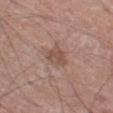<record>
<biopsy_status>not biopsied; imaged during a skin examination</biopsy_status>
<lesion_size>
  <long_diameter_mm_approx>3.0</long_diameter_mm_approx>
</lesion_size>
<automated_metrics>
  <vs_skin_darker_L>8.0</vs_skin_darker_L>
  <border_irregularity_0_10>3.0</border_irregularity_0_10>
  <color_variation_0_10>2.0</color_variation_0_10>
</automated_metrics>
<patient>
  <sex>male</sex>
  <age_approx>75</age_approx>
</patient>
<lighting>white-light</lighting>
<image>
  <source>total-body photography crop</source>
  <field_of_view_mm>15</field_of_view_mm>
</image>
<site>leg</site>
</record>